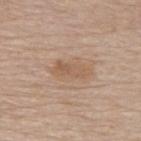biopsy status: imaged on a skin check; not biopsied
image: ~15 mm tile from a whole-body skin photo
anatomic site: the upper back
size: ≈4 mm
lighting: white-light
subject: male, aged around 75